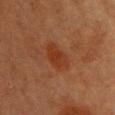{
  "patient": {
    "sex": "female",
    "age_approx": 40
  },
  "site": "chest",
  "image": {
    "source": "total-body photography crop",
    "field_of_view_mm": 15
  },
  "lesion_size": {
    "long_diameter_mm_approx": 4.0
  },
  "lighting": "cross-polarized"
}